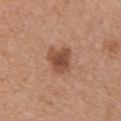Context:
On the chest. Cropped from a total-body skin-imaging series; the visible field is about 15 mm. A female subject, aged 33 to 37. Automated tile analysis of the lesion measured a footprint of about 8.5 mm² and an eccentricity of roughly 0.5. The software also gave border irregularity of about 3 on a 0–10 scale, a within-lesion color-variation index near 3.5/10, and radial color variation of about 1.